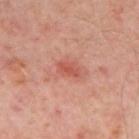biopsy status=no biopsy performed (imaged during a skin exam)
lesion diameter=about 2.5 mm
subject=in their mid-50s
automated metrics=a nevus-likeness score of about 0/100 and a lesion-detection confidence of about 100/100
lighting=cross-polarized illumination
acquisition=15 mm crop, total-body photography
site=the back